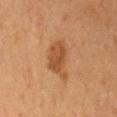location: the abdomen | lesion diameter: ~5 mm (longest diameter) | patient: male, roughly 60 years of age | image: ~15 mm tile from a whole-body skin photo.